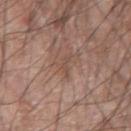<record>
<biopsy_status>not biopsied; imaged during a skin examination</biopsy_status>
<patient>
  <sex>male</sex>
  <age_approx>60</age_approx>
</patient>
<lesion_size>
  <long_diameter_mm_approx>3.0</long_diameter_mm_approx>
</lesion_size>
<lighting>white-light</lighting>
<site>left forearm</site>
<image>
  <source>total-body photography crop</source>
  <field_of_view_mm>15</field_of_view_mm>
</image>
</record>